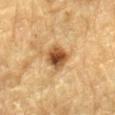Q: Automated lesion metrics?
A: a footprint of about 7 mm² and a shape-asymmetry score of about 0.2 (0 = symmetric); about 15 CIELAB-L* units darker than the surrounding skin and a normalized lesion–skin contrast near 11; a nevus-likeness score of about 95/100 and a lesion-detection confidence of about 100/100
Q: What is the anatomic site?
A: the back
Q: How large is the lesion?
A: ~3 mm (longest diameter)
Q: How was the tile lit?
A: cross-polarized illumination
Q: What kind of image is this?
A: 15 mm crop, total-body photography
Q: Who is the patient?
A: male, aged approximately 85 or older